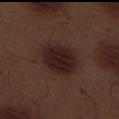The lesion was tiled from a total-body skin photograph and was not biopsied.
A male subject, aged around 70.
The lesion is located on the left thigh.
The tile uses white-light illumination.
A 15 mm close-up tile from a total-body photography series done for melanoma screening.